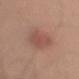Q: Is there a histopathology result?
A: imaged on a skin check; not biopsied